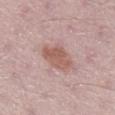notes — no biopsy performed (imaged during a skin exam); patient — male, approximately 50 years of age; lighting — white-light illumination; body site — the right lower leg; image-analysis metrics — a classifier nevus-likeness of about 70/100 and a detector confidence of about 100 out of 100 that the crop contains a lesion; image source — ~15 mm crop, total-body skin-cancer survey; lesion diameter — about 4.5 mm.